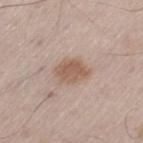Q: Was this lesion biopsied?
A: catalogued during a skin exam; not biopsied
Q: Who is the patient?
A: male, aged approximately 55
Q: Lesion location?
A: the leg
Q: What kind of image is this?
A: 15 mm crop, total-body photography